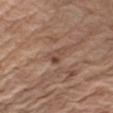This image is a 15 mm lesion crop taken from a total-body photograph. Measured at roughly 2.5 mm in maximum diameter. Captured under white-light illumination. The lesion is on the right upper arm. The subject is a male aged 68–72.